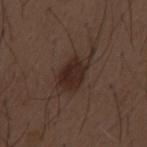| key | value |
|---|---|
| workup | total-body-photography surveillance lesion; no biopsy |
| patient | male, aged approximately 50 |
| lesion diameter | ~6 mm (longest diameter) |
| image | ~15 mm crop, total-body skin-cancer survey |
| body site | the mid back |
| image-analysis metrics | an eccentricity of roughly 0.8 and two-axis asymmetry of about 0.3; an average lesion color of about L≈26 a*≈15 b*≈20 (CIELAB), roughly 8 lightness units darker than nearby skin, and a lesion-to-skin contrast of about 8.5 (normalized; higher = more distinct); a nevus-likeness score of about 95/100 and lesion-presence confidence of about 100/100 |